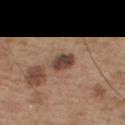The lesion was photographed on a routine skin check and not biopsied; there is no pathology result. The total-body-photography lesion software estimated an area of roughly 6 mm², an outline eccentricity of about 0.7 (0 = round, 1 = elongated), and a symmetry-axis asymmetry near 0.15. The analysis additionally found a mean CIELAB color near L≈44 a*≈18 b*≈25 and a normalized lesion–skin contrast near 10.5. And it measured a border-irregularity rating of about 1.5/10, internal color variation of about 5.5 on a 0–10 scale, and radial color variation of about 2. The software also gave an automated nevus-likeness rating near 30 out of 100 and lesion-presence confidence of about 100/100. The patient is a male aged around 55. A close-up tile cropped from a whole-body skin photograph, about 15 mm across. From the front of the torso. Captured under white-light illumination. The lesion's longest dimension is about 3.5 mm.